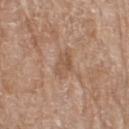Impression:
Captured during whole-body skin photography for melanoma surveillance; the lesion was not biopsied.
Acquisition and patient details:
A female subject, roughly 75 years of age. The recorded lesion diameter is about 3.5 mm. Located on the leg. A 15 mm crop from a total-body photograph taken for skin-cancer surveillance. The total-body-photography lesion software estimated an average lesion color of about L≈54 a*≈19 b*≈30 (CIELAB) and a normalized border contrast of about 5.5. The analysis additionally found a border-irregularity rating of about 2.5/10 and peripheral color asymmetry of about 1.